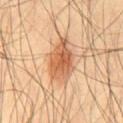<record>
<biopsy_status>not biopsied; imaged during a skin examination</biopsy_status>
<site>front of the torso</site>
<automated_metrics>
  <area_mm2_approx>14.0</area_mm2_approx>
  <eccentricity>0.75</eccentricity>
  <shape_asymmetry>0.2</shape_asymmetry>
  <cielab_L>59</cielab_L>
  <cielab_a>24</cielab_a>
  <cielab_b>37</cielab_b>
  <vs_skin_darker_L>12.0</vs_skin_darker_L>
  <vs_skin_contrast_norm>8.0</vs_skin_contrast_norm>
  <nevus_likeness_0_100>100</nevus_likeness_0_100>
  <lesion_detection_confidence_0_100>100</lesion_detection_confidence_0_100>
</automated_metrics>
<image>
  <source>total-body photography crop</source>
  <field_of_view_mm>15</field_of_view_mm>
</image>
<patient>
  <sex>male</sex>
  <age_approx>55</age_approx>
</patient>
<lesion_size>
  <long_diameter_mm_approx>5.0</long_diameter_mm_approx>
</lesion_size>
</record>